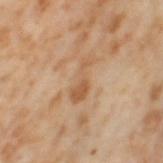No biopsy was performed on this lesion — it was imaged during a full skin examination and was not determined to be concerning.
Cropped from a whole-body photographic skin survey; the tile spans about 15 mm.
Automated image analysis of the tile measured border irregularity of about 7 on a 0–10 scale, a within-lesion color-variation index near 1/10, and peripheral color asymmetry of about 0.5. The analysis additionally found a lesion-detection confidence of about 100/100.
The tile uses cross-polarized illumination.
From the left thigh.
The subject is a female aged 53–57.